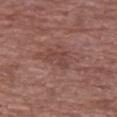Notes:
- biopsy status: imaged on a skin check; not biopsied
- anatomic site: the upper back
- acquisition: 15 mm crop, total-body photography
- patient: female, approximately 75 years of age
- tile lighting: white-light illumination
- automated metrics: a lesion color around L≈43 a*≈21 b*≈23 in CIELAB, about 7 CIELAB-L* units darker than the surrounding skin, and a lesion-to-skin contrast of about 5.5 (normalized; higher = more distinct)